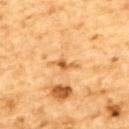<case>
<biopsy_status>not biopsied; imaged during a skin examination</biopsy_status>
<patient>
  <sex>male</sex>
  <age_approx>85+</age_approx>
</patient>
<lighting>cross-polarized</lighting>
<site>upper back</site>
<image>
  <source>total-body photography crop</source>
  <field_of_view_mm>15</field_of_view_mm>
</image>
</case>